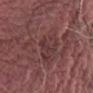{
  "biopsy_status": "not biopsied; imaged during a skin examination",
  "automated_metrics": {
    "cielab_L": 34,
    "cielab_a": 21,
    "cielab_b": 17,
    "vs_skin_darker_L": 5.0,
    "vs_skin_contrast_norm": 5.0,
    "color_variation_0_10": 0.0,
    "nevus_likeness_0_100": 0
  },
  "lighting": "white-light",
  "image": {
    "source": "total-body photography crop",
    "field_of_view_mm": 15
  },
  "patient": {
    "sex": "male",
    "age_approx": 60
  },
  "site": "left thigh",
  "lesion_size": {
    "long_diameter_mm_approx": 3.5
  }
}